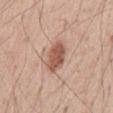Clinical impression:
No biopsy was performed on this lesion — it was imaged during a full skin examination and was not determined to be concerning.
Image and clinical context:
Approximately 4 mm at its widest. Automated tile analysis of the lesion measured a color-variation rating of about 2.5/10 and a peripheral color-asymmetry measure near 1. The analysis additionally found a classifier nevus-likeness of about 95/100 and lesion-presence confidence of about 100/100. This image is a 15 mm lesion crop taken from a total-body photograph. The lesion is on the abdomen. The tile uses white-light illumination. A male patient aged around 55.